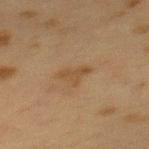This lesion was catalogued during total-body skin photography and was not selected for biopsy.
A 15 mm crop from a total-body photograph taken for skin-cancer surveillance.
Approximately 3 mm at its widest.
The patient is a female aged around 40.
This is a cross-polarized tile.
From the upper back.